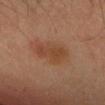Assessment:
This lesion was catalogued during total-body skin photography and was not selected for biopsy.
Image and clinical context:
A male patient, aged 33–37. A region of skin cropped from a whole-body photographic capture, roughly 15 mm wide. The lesion is located on the head or neck. Measured at roughly 4.5 mm in maximum diameter. The tile uses cross-polarized illumination.